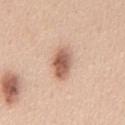Impression: Recorded during total-body skin imaging; not selected for excision or biopsy. Acquisition and patient details: The patient is a male in their mid- to late 40s. A roughly 15 mm field-of-view crop from a total-body skin photograph. From the chest.